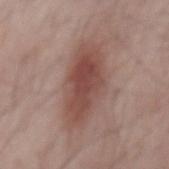Recorded during total-body skin imaging; not selected for excision or biopsy. Imaged with white-light lighting. A lesion tile, about 15 mm wide, cut from a 3D total-body photograph. A male subject roughly 65 years of age. The lesion is located on the back.